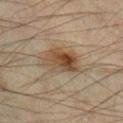Imaged during a routine full-body skin examination; the lesion was not biopsied and no histopathology is available.
The lesion is located on the leg.
A lesion tile, about 15 mm wide, cut from a 3D total-body photograph.
A male patient roughly 55 years of age.